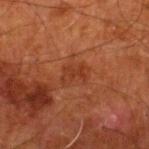Q: Was a biopsy performed?
A: imaged on a skin check; not biopsied